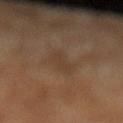{"biopsy_status": "not biopsied; imaged during a skin examination", "site": "left lower leg", "patient": {"sex": "male", "age_approx": 70}, "lesion_size": {"long_diameter_mm_approx": 5.5}, "image": {"source": "total-body photography crop", "field_of_view_mm": 15}, "lighting": "cross-polarized"}